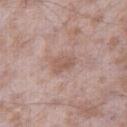| key | value |
|---|---|
| notes | no biopsy performed (imaged during a skin exam) |
| lesion size | ~3 mm (longest diameter) |
| automated metrics | a lesion color around L≈56 a*≈19 b*≈25 in CIELAB, about 8 CIELAB-L* units darker than the surrounding skin, and a normalized border contrast of about 6; a border-irregularity rating of about 3/10, a color-variation rating of about 2/10, and radial color variation of about 1 |
| patient | male, aged around 45 |
| body site | the right thigh |
| lighting | white-light illumination |
| imaging modality | ~15 mm crop, total-body skin-cancer survey |